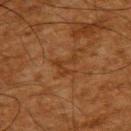No biopsy was performed on this lesion — it was imaged during a full skin examination and was not determined to be concerning. A male subject in their mid-60s. Cropped from a whole-body photographic skin survey; the tile spans about 15 mm. The recorded lesion diameter is about 3 mm. The lesion is on the upper back. Captured under cross-polarized illumination.